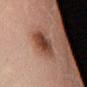Notes:
* notes: no biopsy performed (imaged during a skin exam)
* image: total-body-photography crop, ~15 mm field of view
* location: the left lower leg
* automated lesion analysis: a footprint of about 10 mm² and a symmetry-axis asymmetry near 0.15; a border-irregularity rating of about 2/10, a within-lesion color-variation index near 6/10, and radial color variation of about 2
* subject: female, roughly 55 years of age
* lesion diameter: ≈3.5 mm